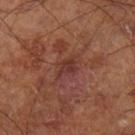• notes: no biopsy performed (imaged during a skin exam)
• illumination: cross-polarized
• patient: male, about 70 years old
• image source: ~15 mm tile from a whole-body skin photo
• size: about 3 mm
• site: the left lower leg